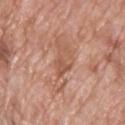Q: Is there a histopathology result?
A: catalogued during a skin exam; not biopsied
Q: What are the patient's age and sex?
A: male, roughly 70 years of age
Q: What lighting was used for the tile?
A: white-light
Q: Lesion size?
A: ~6.5 mm (longest diameter)
Q: Where on the body is the lesion?
A: the chest
Q: How was this image acquired?
A: total-body-photography crop, ~15 mm field of view